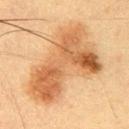  biopsy_status: not biopsied; imaged during a skin examination
  automated_metrics:
    area_mm2_approx: 47.0
    eccentricity: 0.9
    shape_asymmetry: 0.5
    border_irregularity_0_10: 6.0
    color_variation_0_10: 8.0
    peripheral_color_asymmetry: 3.0
    nevus_likeness_0_100: 5
    lesion_detection_confidence_0_100: 100
  image:
    source: total-body photography crop
    field_of_view_mm: 15
  lighting: cross-polarized
  lesion_size:
    long_diameter_mm_approx: 12.0
  site: left upper arm
  patient:
    sex: male
    age_approx: 55